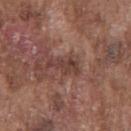biopsy status = total-body-photography surveillance lesion; no biopsy | anatomic site = the chest | image = 15 mm crop, total-body photography | illumination = white-light illumination | diameter = about 3.5 mm | subject = male, about 75 years old.